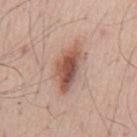| key | value |
|---|---|
| notes | catalogued during a skin exam; not biopsied |
| site | the mid back |
| automated lesion analysis | a lesion color around L≈54 a*≈22 b*≈26 in CIELAB, a lesion–skin lightness drop of about 14, and a normalized border contrast of about 9.5; an automated nevus-likeness rating near 100 out of 100 and lesion-presence confidence of about 100/100 |
| lesion diameter | about 6.5 mm |
| imaging modality | 15 mm crop, total-body photography |
| subject | male, about 55 years old |
| tile lighting | white-light |